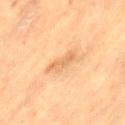Impression:
The lesion was tiled from a total-body skin photograph and was not biopsied.
Acquisition and patient details:
A female patient, approximately 70 years of age. A 15 mm close-up extracted from a 3D total-body photography capture. Longest diameter approximately 3.5 mm. The lesion is on the left thigh. The total-body-photography lesion software estimated a mean CIELAB color near L≈68 a*≈22 b*≈41 and a normalized border contrast of about 5.5. The software also gave border irregularity of about 4 on a 0–10 scale, a color-variation rating of about 2/10, and peripheral color asymmetry of about 0.5. Imaged with cross-polarized lighting.